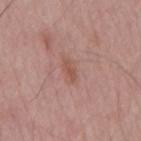| feature | finding |
|---|---|
| workup | total-body-photography surveillance lesion; no biopsy |
| patient | male, aged 53 to 57 |
| image | ~15 mm crop, total-body skin-cancer survey |
| diameter | about 3 mm |
| lighting | white-light |